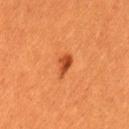{"biopsy_status": "not biopsied; imaged during a skin examination", "site": "left thigh", "image": {"source": "total-body photography crop", "field_of_view_mm": 15}, "lesion_size": {"long_diameter_mm_approx": 2.5}, "lighting": "cross-polarized", "patient": {"sex": "female", "age_approx": 30}}